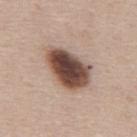The lesion was photographed on a routine skin check and not biopsied; there is no pathology result. About 6 mm across. This image is a 15 mm lesion crop taken from a total-body photograph. An algorithmic analysis of the crop reported border irregularity of about 2 on a 0–10 scale, a color-variation rating of about 7/10, and a peripheral color-asymmetry measure near 2. And it measured an automated nevus-likeness rating near 95 out of 100. Located on the mid back. A male patient aged approximately 45. Imaged with white-light lighting.